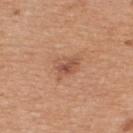This lesion was catalogued during total-body skin photography and was not selected for biopsy. A close-up tile cropped from a whole-body skin photograph, about 15 mm across. The lesion is on the upper back. This is a white-light tile. About 3.5 mm across. The subject is a female aged 28 to 32.